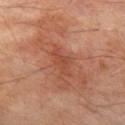Recorded during total-body skin imaging; not selected for excision or biopsy. A lesion tile, about 15 mm wide, cut from a 3D total-body photograph. On the leg. The patient is a male about 70 years old.